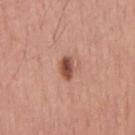The lesion was photographed on a routine skin check and not biopsied; there is no pathology result. An algorithmic analysis of the crop reported an automated nevus-likeness rating near 95 out of 100 and a lesion-detection confidence of about 100/100. The subject is a male aged around 45. This is a white-light tile. A region of skin cropped from a whole-body photographic capture, roughly 15 mm wide. The lesion's longest dimension is about 2.5 mm. From the upper back.